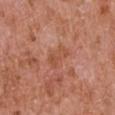Recorded during total-body skin imaging; not selected for excision or biopsy. A close-up tile cropped from a whole-body skin photograph, about 15 mm across. The lesion is located on the arm. Automated image analysis of the tile measured a border-irregularity index near 3.5/10 and a within-lesion color-variation index near 3/10. It also reported a nevus-likeness score of about 0/100 and lesion-presence confidence of about 100/100. The subject is a male in their mid- to late 60s.